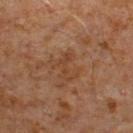Clinical impression:
The lesion was photographed on a routine skin check and not biopsied; there is no pathology result.
Context:
The tile uses cross-polarized illumination. The lesion is located on the right lower leg. A male patient in their 60s. The recorded lesion diameter is about 3.5 mm. A 15 mm close-up tile from a total-body photography series done for melanoma screening.